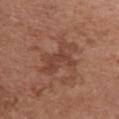| feature | finding |
|---|---|
| workup | total-body-photography surveillance lesion; no biopsy |
| subject | female, aged 73 to 77 |
| lighting | white-light illumination |
| lesion size | about 6 mm |
| anatomic site | the front of the torso |
| imaging modality | ~15 mm tile from a whole-body skin photo |